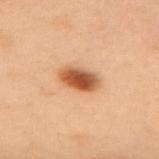Part of a total-body skin-imaging series; this lesion was reviewed on a skin check and was not flagged for biopsy. Imaged with cross-polarized lighting. From the back. Approximately 4.5 mm at its widest. A roughly 15 mm field-of-view crop from a total-body skin photograph. A female subject, aged around 55. An algorithmic analysis of the crop reported an eccentricity of roughly 0.85. It also reported an average lesion color of about L≈46 a*≈22 b*≈33 (CIELAB) and a normalized lesion–skin contrast near 10.5.